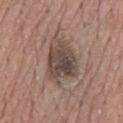Case summary:
– biopsy status — catalogued during a skin exam; not biopsied
– patient — male, approximately 75 years of age
– illumination — white-light illumination
– acquisition — ~15 mm crop, total-body skin-cancer survey
– lesion diameter — about 6 mm
– body site — the mid back